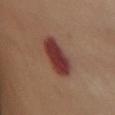follow-up — total-body-photography surveillance lesion; no biopsy
lighting — cross-polarized illumination
imaging modality — ~15 mm tile from a whole-body skin photo
patient — female, aged around 60
body site — the chest
TBP lesion metrics — a mean CIELAB color near L≈31 a*≈23 b*≈20; a nevus-likeness score of about 90/100 and a detector confidence of about 100 out of 100 that the crop contains a lesion